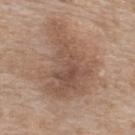{"biopsy_status": "not biopsied; imaged during a skin examination", "patient": {"sex": "female", "age_approx": 75}, "lighting": "white-light", "automated_metrics": {"border_irregularity_0_10": 6.0, "color_variation_0_10": 4.5, "peripheral_color_asymmetry": 1.5, "nevus_likeness_0_100": 0, "lesion_detection_confidence_0_100": 100}, "site": "upper back", "image": {"source": "total-body photography crop", "field_of_view_mm": 15}}